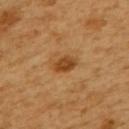site — the upper back
patient — female, about 55 years old
imaging modality — ~15 mm crop, total-body skin-cancer survey